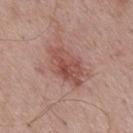<case>
<biopsy_status>not biopsied; imaged during a skin examination</biopsy_status>
<image>
  <source>total-body photography crop</source>
  <field_of_view_mm>15</field_of_view_mm>
</image>
<lesion_size>
  <long_diameter_mm_approx>5.5</long_diameter_mm_approx>
</lesion_size>
<lighting>white-light</lighting>
<patient>
  <sex>male</sex>
  <age_approx>70</age_approx>
</patient>
<site>mid back</site>
<automated_metrics>
  <eccentricity>0.85</eccentricity>
  <shape_asymmetry>0.25</shape_asymmetry>
  <cielab_L>51</cielab_L>
  <cielab_a>24</cielab_a>
  <cielab_b>25</cielab_b>
  <vs_skin_darker_L>10.0</vs_skin_darker_L>
  <vs_skin_contrast_norm>7.0</vs_skin_contrast_norm>
  <border_irregularity_0_10>3.5</border_irregularity_0_10>
  <color_variation_0_10>4.5</color_variation_0_10>
  <peripheral_color_asymmetry>1.5</peripheral_color_asymmetry>
</automated_metrics>
</case>